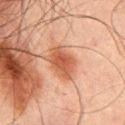| feature | finding |
|---|---|
| notes | total-body-photography surveillance lesion; no biopsy |
| lighting | cross-polarized |
| anatomic site | the mid back |
| patient | male, aged approximately 65 |
| TBP lesion metrics | roughly 10 lightness units darker than nearby skin; a border-irregularity rating of about 2.5/10, a within-lesion color-variation index near 3.5/10, and radial color variation of about 1 |
| size | about 4 mm |
| image source | ~15 mm crop, total-body skin-cancer survey |